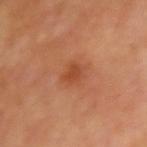The lesion was photographed on a routine skin check and not biopsied; there is no pathology result. The lesion is located on the right upper arm. A female subject, aged 53–57. The tile uses cross-polarized illumination. Approximately 2.5 mm at its widest. Cropped from a total-body skin-imaging series; the visible field is about 15 mm.